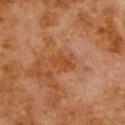This lesion was catalogued during total-body skin photography and was not selected for biopsy. From the upper back. This is a cross-polarized tile. A male subject, aged 78 to 82. The lesion's longest dimension is about 3 mm. A 15 mm close-up extracted from a 3D total-body photography capture.